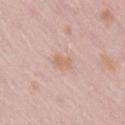workup: no biopsy performed (imaged during a skin exam) | anatomic site: the abdomen | lighting: white-light illumination | patient: male, approximately 60 years of age | diameter: ≈2.5 mm | acquisition: total-body-photography crop, ~15 mm field of view | TBP lesion metrics: a mean CIELAB color near L≈66 a*≈18 b*≈29 and roughly 6 lightness units darker than nearby skin; a border-irregularity rating of about 2.5/10, a within-lesion color-variation index near 2/10, and radial color variation of about 0.5.